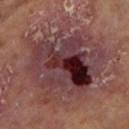notes — no biopsy performed (imaged during a skin exam) | lighting — cross-polarized | lesion size — about 11 mm | patient — male, aged around 70 | body site — the left lower leg | acquisition — total-body-photography crop, ~15 mm field of view.